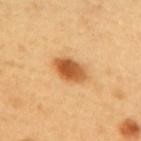The lesion was tiled from a total-body skin photograph and was not biopsied. The lesion is located on the upper back. This is a cross-polarized tile. A lesion tile, about 15 mm wide, cut from a 3D total-body photograph. A female patient, in their mid-50s. Longest diameter approximately 4.5 mm.